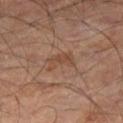{
  "biopsy_status": "not biopsied; imaged during a skin examination",
  "image": {
    "source": "total-body photography crop",
    "field_of_view_mm": 15
  },
  "site": "leg",
  "lighting": "cross-polarized",
  "automated_metrics": {
    "cielab_L": 42,
    "cielab_a": 18,
    "cielab_b": 27,
    "vs_skin_darker_L": 6.0,
    "vs_skin_contrast_norm": 5.5
  },
  "patient": {
    "sex": "male",
    "age_approx": 55
  },
  "lesion_size": {
    "long_diameter_mm_approx": 3.5
  }
}